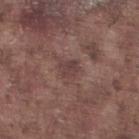Q: Was a biopsy performed?
A: catalogued during a skin exam; not biopsied
Q: How was the tile lit?
A: white-light illumination
Q: What did automated image analysis measure?
A: a lesion color around L≈41 a*≈18 b*≈19 in CIELAB and about 7 CIELAB-L* units darker than the surrounding skin; border irregularity of about 3.5 on a 0–10 scale and internal color variation of about 2 on a 0–10 scale
Q: What is the imaging modality?
A: total-body-photography crop, ~15 mm field of view
Q: What are the patient's age and sex?
A: male, in their mid- to late 70s
Q: What is the anatomic site?
A: the right lower leg